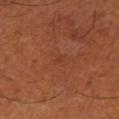  biopsy_status: not biopsied; imaged during a skin examination
  patient:
    sex: male
    age_approx: 50
  site: leg
  automated_metrics:
    area_mm2_approx: 1.0
    eccentricity: 0.8
  image:
    source: total-body photography crop
    field_of_view_mm: 15
  lighting: cross-polarized
  lesion_size:
    long_diameter_mm_approx: 1.0